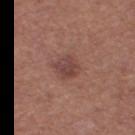notes: no biopsy performed (imaged during a skin exam)
image source: ~15 mm tile from a whole-body skin photo
image-analysis metrics: a lesion area of about 6 mm² and an outline eccentricity of about 0.6 (0 = round, 1 = elongated); an automated nevus-likeness rating near 50 out of 100 and lesion-presence confidence of about 100/100
lesion diameter: ~3.5 mm (longest diameter)
patient: female, aged 43 to 47
site: the right thigh
illumination: white-light illumination